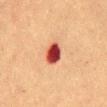This lesion was catalogued during total-body skin photography and was not selected for biopsy. The tile uses cross-polarized illumination. The total-body-photography lesion software estimated a footprint of about 6.5 mm², a shape eccentricity near 0.6, and a shape-asymmetry score of about 0.15 (0 = symmetric). The analysis additionally found an average lesion color of about L≈41 a*≈31 b*≈28 (CIELAB), roughly 18 lightness units darker than nearby skin, and a normalized lesion–skin contrast near 14. The analysis additionally found a border-irregularity rating of about 1.5/10, a color-variation rating of about 6/10, and radial color variation of about 2. A male subject, aged 48 to 52. On the abdomen. A roughly 15 mm field-of-view crop from a total-body skin photograph.